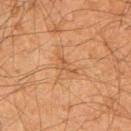Assessment: The lesion was photographed on a routine skin check and not biopsied; there is no pathology result. Context: About 3 mm across. The subject is a male approximately 65 years of age. A 15 mm close-up tile from a total-body photography series done for melanoma screening. On the left upper arm. Captured under cross-polarized illumination.